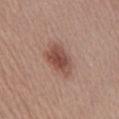Q: Illumination type?
A: white-light illumination
Q: Lesion location?
A: the front of the torso
Q: What did automated image analysis measure?
A: a footprint of about 9.5 mm², a shape eccentricity near 0.75, and a shape-asymmetry score of about 0.25 (0 = symmetric); an average lesion color of about L≈49 a*≈23 b*≈25 (CIELAB) and roughly 11 lightness units darker than nearby skin; a border-irregularity rating of about 2.5/10, internal color variation of about 3.5 on a 0–10 scale, and a peripheral color-asymmetry measure near 1; a lesion-detection confidence of about 100/100
Q: How large is the lesion?
A: ~4.5 mm (longest diameter)
Q: What is the imaging modality?
A: 15 mm crop, total-body photography
Q: Who is the patient?
A: male, in their mid- to late 50s